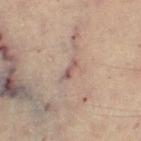{"biopsy_status": "not biopsied; imaged during a skin examination", "site": "left thigh", "automated_metrics": {"border_irregularity_0_10": 5.0, "color_variation_0_10": 0.0}, "image": {"source": "total-body photography crop", "field_of_view_mm": 15}, "patient": {"sex": "female", "age_approx": 60}, "lesion_size": {"long_diameter_mm_approx": 2.5}, "lighting": "cross-polarized"}